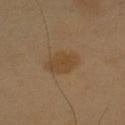Image and clinical context: A 15 mm crop from a total-body photograph taken for skin-cancer surveillance. This is a cross-polarized tile. From the left upper arm. A male patient aged 58–62. The lesion-visualizer software estimated an area of roughly 8.5 mm² and an eccentricity of roughly 0.75. The analysis additionally found a nevus-likeness score of about 40/100 and a lesion-detection confidence of about 100/100.